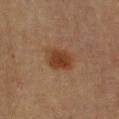follow-up: total-body-photography surveillance lesion; no biopsy
image source: total-body-photography crop, ~15 mm field of view
lesion size: ~3.5 mm (longest diameter)
subject: male, aged around 65
site: the right upper arm
tile lighting: cross-polarized